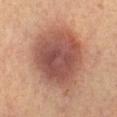Captured during whole-body skin photography for melanoma surveillance; the lesion was not biopsied.